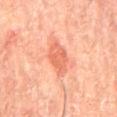Measured at roughly 4.5 mm in maximum diameter. Automated image analysis of the tile measured an average lesion color of about L≈66 a*≈32 b*≈37 (CIELAB), roughly 10 lightness units darker than nearby skin, and a lesion-to-skin contrast of about 6.5 (normalized; higher = more distinct). And it measured a border-irregularity index near 2.5/10, a color-variation rating of about 2.5/10, and radial color variation of about 1. A male subject, aged around 65. A region of skin cropped from a whole-body photographic capture, roughly 15 mm wide. Located on the mid back.